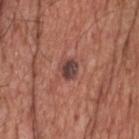biopsy status: no biopsy performed (imaged during a skin exam) | image source: ~15 mm tile from a whole-body skin photo | tile lighting: white-light illumination | location: the head or neck | image-analysis metrics: a border-irregularity rating of about 2.5/10, a within-lesion color-variation index near 4.5/10, and radial color variation of about 1.5 | patient: male, aged approximately 60.